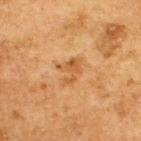Assessment: The lesion was tiled from a total-body skin photograph and was not biopsied. Context: The lesion is located on the upper back. A 15 mm close-up extracted from a 3D total-body photography capture. Automated image analysis of the tile measured a footprint of about 6 mm², an eccentricity of roughly 0.65, and a shape-asymmetry score of about 0.45 (0 = symmetric). It also reported a mean CIELAB color near L≈46 a*≈19 b*≈36, a lesion–skin lightness drop of about 7, and a lesion-to-skin contrast of about 6 (normalized; higher = more distinct). The analysis additionally found a border-irregularity rating of about 5.5/10, internal color variation of about 2.5 on a 0–10 scale, and a peripheral color-asymmetry measure near 1. A male patient, aged around 75. The tile uses cross-polarized illumination.